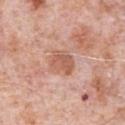notes — no biopsy performed (imaged during a skin exam)
illumination — white-light illumination
patient — male, aged approximately 80
image — ~15 mm tile from a whole-body skin photo
diameter — ~3 mm (longest diameter)
image-analysis metrics — a lesion color around L≈58 a*≈24 b*≈30 in CIELAB and a lesion–skin lightness drop of about 10; border irregularity of about 2.5 on a 0–10 scale, internal color variation of about 3 on a 0–10 scale, and radial color variation of about 1; an automated nevus-likeness rating near 0 out of 100 and a detector confidence of about 100 out of 100 that the crop contains a lesion
anatomic site — the chest